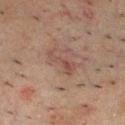biopsy_status: not biopsied; imaged during a skin examination
lesion_size:
  long_diameter_mm_approx: 4.0
patient:
  sex: male
  age_approx: 50
image:
  source: total-body photography crop
  field_of_view_mm: 15
lighting: cross-polarized
site: chest